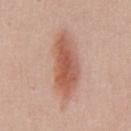Captured during whole-body skin photography for melanoma surveillance; the lesion was not biopsied. The tile uses white-light illumination. Cropped from a total-body skin-imaging series; the visible field is about 15 mm. Automated tile analysis of the lesion measured a classifier nevus-likeness of about 100/100 and lesion-presence confidence of about 100/100. A male patient, aged 58–62. The lesion is located on the abdomen. Longest diameter approximately 7.5 mm.